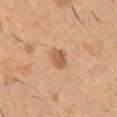Impression: No biopsy was performed on this lesion — it was imaged during a full skin examination and was not determined to be concerning. Context: A close-up tile cropped from a whole-body skin photograph, about 15 mm across. Located on the left upper arm. A male patient in their 40s. An algorithmic analysis of the crop reported an area of roughly 4.5 mm² and a symmetry-axis asymmetry near 0.25. The software also gave a nevus-likeness score of about 70/100.